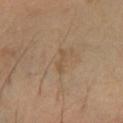workup: total-body-photography surveillance lesion; no biopsy
anatomic site: the left lower leg
subject: male, aged approximately 45
image source: 15 mm crop, total-body photography